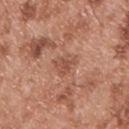biopsy status: no biopsy performed (imaged during a skin exam)
patient: male, roughly 55 years of age
image: total-body-photography crop, ~15 mm field of view
lesion size: about 2.5 mm
TBP lesion metrics: a footprint of about 4.5 mm², an eccentricity of roughly 0.4, and a symmetry-axis asymmetry near 0.35; border irregularity of about 3.5 on a 0–10 scale, internal color variation of about 2.5 on a 0–10 scale, and a peripheral color-asymmetry measure near 1
body site: the upper back